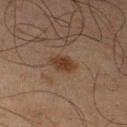Q: Was a biopsy performed?
A: no biopsy performed (imaged during a skin exam)
Q: What is the lesion's diameter?
A: ≈3.5 mm
Q: Lesion location?
A: the left lower leg
Q: What are the patient's age and sex?
A: male, in their mid- to late 60s
Q: What did automated image analysis measure?
A: an eccentricity of roughly 0.8; a mean CIELAB color near L≈30 a*≈15 b*≈25, about 8 CIELAB-L* units darker than the surrounding skin, and a normalized border contrast of about 8.5
Q: What kind of image is this?
A: 15 mm crop, total-body photography